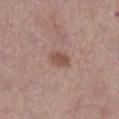follow-up: total-body-photography surveillance lesion; no biopsy | illumination: white-light | lesion diameter: ~3 mm (longest diameter) | patient: female, approximately 65 years of age | image-analysis metrics: a footprint of about 4.5 mm² and an outline eccentricity of about 0.8 (0 = round, 1 = elongated); a border-irregularity rating of about 1.5/10, a color-variation rating of about 2/10, and radial color variation of about 0.5 | body site: the leg | image: 15 mm crop, total-body photography.